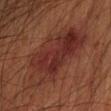{"biopsy_status": "not biopsied; imaged during a skin examination", "site": "right forearm", "image": {"source": "total-body photography crop", "field_of_view_mm": 15}, "lesion_size": {"long_diameter_mm_approx": 8.5}, "patient": {"sex": "male", "age_approx": 65}}